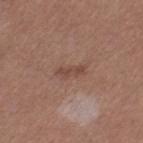| field | value |
|---|---|
| notes | no biopsy performed (imaged during a skin exam) |
| patient | female, aged 43 to 47 |
| image | total-body-photography crop, ~15 mm field of view |
| site | the left thigh |